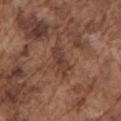anatomic site = the arm; lighting = white-light illumination; patient = male, in their mid- to late 70s; image source = ~15 mm tile from a whole-body skin photo; size = ~4 mm (longest diameter).